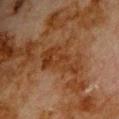workup: catalogued during a skin exam; not biopsied
anatomic site: the upper back
image: 15 mm crop, total-body photography
illumination: cross-polarized illumination
lesion size: ≈6 mm
patient: male, aged approximately 80